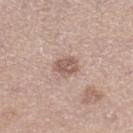– workup · no biopsy performed (imaged during a skin exam)
– lesion diameter · ≈3 mm
– lighting · white-light illumination
– patient · female, aged around 35
– image · ~15 mm crop, total-body skin-cancer survey
– body site · the right lower leg